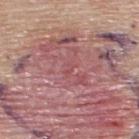  image:
    source: total-body photography crop
    field_of_view_mm: 15
  site: back
  patient:
    sex: male
    age_approx: 55
  lesion_size:
    long_diameter_mm_approx: 2.0
  automated_metrics:
    cielab_L: 51
    cielab_a: 29
    cielab_b: 21
    vs_skin_contrast_norm: 3.5
    lesion_detection_confidence_0_100: 5